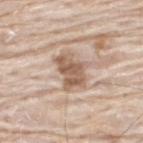follow-up = no biopsy performed (imaged during a skin exam) | patient = male, roughly 75 years of age | site = the upper back | image source = ~15 mm tile from a whole-body skin photo.